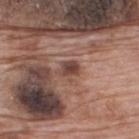Case summary:
- notes: catalogued during a skin exam; not biopsied